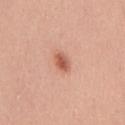Q: How was this image acquired?
A: ~15 mm crop, total-body skin-cancer survey
Q: What are the patient's age and sex?
A: male, aged approximately 30
Q: Where on the body is the lesion?
A: the chest